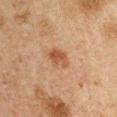Q: Was a biopsy performed?
A: catalogued during a skin exam; not biopsied
Q: What are the patient's age and sex?
A: male, approximately 50 years of age
Q: Illumination type?
A: cross-polarized
Q: How was this image acquired?
A: ~15 mm crop, total-body skin-cancer survey
Q: What is the lesion's diameter?
A: ≈3 mm
Q: What is the anatomic site?
A: the left upper arm
Q: What did automated image analysis measure?
A: a mean CIELAB color near L≈41 a*≈18 b*≈29; a border-irregularity rating of about 2/10 and radial color variation of about 1.5